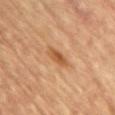Assessment:
Part of a total-body skin-imaging series; this lesion was reviewed on a skin check and was not flagged for biopsy.
Context:
A lesion tile, about 15 mm wide, cut from a 3D total-body photograph. A male subject in their mid-50s. The tile uses cross-polarized illumination. The lesion is on the front of the torso. The recorded lesion diameter is about 3.5 mm.